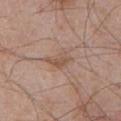| feature | finding |
|---|---|
| notes | imaged on a skin check; not biopsied |
| patient | male, aged around 65 |
| imaging modality | ~15 mm crop, total-body skin-cancer survey |
| location | the chest |